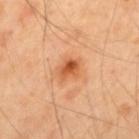Impression: Part of a total-body skin-imaging series; this lesion was reviewed on a skin check and was not flagged for biopsy. Background: About 2.5 mm across. A close-up tile cropped from a whole-body skin photograph, about 15 mm across. The lesion is located on the upper back. The subject is a male about 40 years old. Automated tile analysis of the lesion measured an eccentricity of roughly 0.6 and a symmetry-axis asymmetry near 0.3. The analysis additionally found a lesion–skin lightness drop of about 12 and a normalized lesion–skin contrast near 8. And it measured border irregularity of about 3 on a 0–10 scale, a color-variation rating of about 6.5/10, and radial color variation of about 2.5. And it measured a classifier nevus-likeness of about 75/100 and a detector confidence of about 100 out of 100 that the crop contains a lesion.